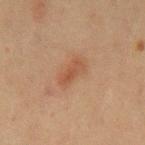This lesion was catalogued during total-body skin photography and was not selected for biopsy. The lesion-visualizer software estimated a mean CIELAB color near L≈42 a*≈19 b*≈29, roughly 6 lightness units darker than nearby skin, and a normalized lesion–skin contrast near 6. It also reported border irregularity of about 3 on a 0–10 scale, a color-variation rating of about 3/10, and a peripheral color-asymmetry measure near 1. The analysis additionally found a classifier nevus-likeness of about 80/100. The lesion is located on the left upper arm. A male subject, aged around 60. A region of skin cropped from a whole-body photographic capture, roughly 15 mm wide. Captured under cross-polarized illumination. The recorded lesion diameter is about 3.5 mm.